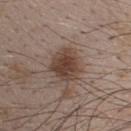Impression: The lesion was tiled from a total-body skin photograph and was not biopsied. Acquisition and patient details: About 4 mm across. The lesion is located on the back. A lesion tile, about 15 mm wide, cut from a 3D total-body photograph. Captured under white-light illumination. A male subject about 50 years old.